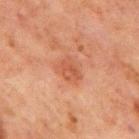Case summary:
– follow-up · catalogued during a skin exam; not biopsied
– automated lesion analysis · an average lesion color of about L≈44 a*≈23 b*≈30 (CIELAB), about 7 CIELAB-L* units darker than the surrounding skin, and a lesion-to-skin contrast of about 5.5 (normalized; higher = more distinct); a border-irregularity rating of about 2.5/10 and internal color variation of about 2.5 on a 0–10 scale; a classifier nevus-likeness of about 45/100 and lesion-presence confidence of about 100/100
– subject · male, in their mid- to late 60s
– image · total-body-photography crop, ~15 mm field of view
– site · the chest